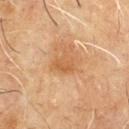Notes:
– biopsy status · imaged on a skin check; not biopsied
– anatomic site · the chest
– illumination · cross-polarized illumination
– lesion diameter · ≈2.5 mm
– patient · male, roughly 60 years of age
– image-analysis metrics · a border-irregularity index near 3/10, internal color variation of about 2 on a 0–10 scale, and a peripheral color-asymmetry measure near 0.5
– imaging modality · 15 mm crop, total-body photography